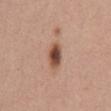<case>
<biopsy_status>not biopsied; imaged during a skin examination</biopsy_status>
<patient>
  <sex>female</sex>
  <age_approx>65</age_approx>
</patient>
<image>
  <source>total-body photography crop</source>
  <field_of_view_mm>15</field_of_view_mm>
</image>
<site>front of the torso</site>
</case>